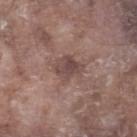{
  "lighting": "white-light",
  "site": "right lower leg",
  "image": {
    "source": "total-body photography crop",
    "field_of_view_mm": 15
  },
  "automated_metrics": {
    "cielab_L": 46,
    "cielab_a": 18,
    "cielab_b": 20,
    "vs_skin_darker_L": 9.0,
    "vs_skin_contrast_norm": 7.0
  },
  "patient": {
    "sex": "male",
    "age_approx": 75
  }
}